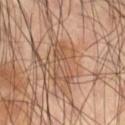| feature | finding |
|---|---|
| follow-up | no biopsy performed (imaged during a skin exam) |
| anatomic site | the right thigh |
| acquisition | 15 mm crop, total-body photography |
| subject | male, aged 58–62 |